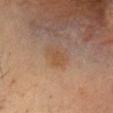This lesion was catalogued during total-body skin photography and was not selected for biopsy. A female patient roughly 45 years of age. A 15 mm close-up extracted from a 3D total-body photography capture. The tile uses cross-polarized illumination. On the head or neck. Longest diameter approximately 2.5 mm.